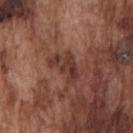Background: The lesion is located on the chest. Captured under white-light illumination. A roughly 15 mm field-of-view crop from a total-body skin photograph. Measured at roughly 4 mm in maximum diameter. Automated image analysis of the tile measured a mean CIELAB color near L≈36 a*≈22 b*≈24 and about 8 CIELAB-L* units darker than the surrounding skin. The analysis additionally found internal color variation of about 4 on a 0–10 scale and peripheral color asymmetry of about 1.5. It also reported an automated nevus-likeness rating near 5 out of 100 and lesion-presence confidence of about 100/100. The patient is a male roughly 75 years of age.